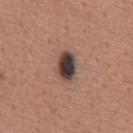An algorithmic analysis of the crop reported a lesion area of about 8 mm², a shape eccentricity near 0.75, and a symmetry-axis asymmetry near 0.15. The analysis additionally found a lesion-to-skin contrast of about 14.5 (normalized; higher = more distinct). And it measured a border-irregularity rating of about 1.5/10. The software also gave a nevus-likeness score of about 100/100 and a lesion-detection confidence of about 100/100. Cropped from a total-body skin-imaging series; the visible field is about 15 mm. The lesion is on the abdomen. The subject is a male in their mid-40s. Measured at roughly 3.5 mm in maximum diameter.